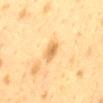Notes:
* image-analysis metrics · an average lesion color of about L≈60 a*≈17 b*≈40 (CIELAB) and a lesion-to-skin contrast of about 7 (normalized; higher = more distinct); border irregularity of about 2.5 on a 0–10 scale, a within-lesion color-variation index near 3/10, and a peripheral color-asymmetry measure near 1
* imaging modality · ~15 mm crop, total-body skin-cancer survey
* subject · female, roughly 40 years of age
* site · the back
* size · about 3 mm